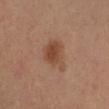Notes:
- notes: no biopsy performed (imaged during a skin exam)
- anatomic site: the right forearm
- imaging modality: 15 mm crop, total-body photography
- subject: female, aged 43 to 47
- illumination: cross-polarized illumination